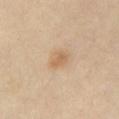workup: catalogued during a skin exam; not biopsied | diameter: ~2.5 mm (longest diameter) | tile lighting: cross-polarized illumination | patient: female, about 45 years old | location: the right thigh | imaging modality: ~15 mm crop, total-body skin-cancer survey.